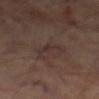Imaged during a routine full-body skin examination; the lesion was not biopsied and no histopathology is available. Captured under cross-polarized illumination. On the leg. A 15 mm close-up tile from a total-body photography series done for melanoma screening. The patient is a male aged 68–72.